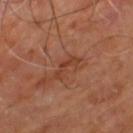No biopsy was performed on this lesion — it was imaged during a full skin examination and was not determined to be concerning.
The subject is aged approximately 65.
The lesion is on the left thigh.
The lesion's longest dimension is about 3.5 mm.
A region of skin cropped from a whole-body photographic capture, roughly 15 mm wide.
The total-body-photography lesion software estimated an area of roughly 3 mm² and an outline eccentricity of about 0.95 (0 = round, 1 = elongated). It also reported internal color variation of about 0 on a 0–10 scale. The software also gave a nevus-likeness score of about 0/100 and lesion-presence confidence of about 100/100.